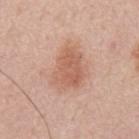| field | value |
|---|---|
| follow-up | imaged on a skin check; not biopsied |
| illumination | white-light |
| automated lesion analysis | a footprint of about 12 mm², an outline eccentricity of about 0.7 (0 = round, 1 = elongated), and two-axis asymmetry of about 0.25; a border-irregularity rating of about 3/10 and a within-lesion color-variation index near 2.5/10; a detector confidence of about 100 out of 100 that the crop contains a lesion |
| size | ~4.5 mm (longest diameter) |
| subject | male, aged around 65 |
| imaging modality | ~15 mm crop, total-body skin-cancer survey |
| site | the mid back |